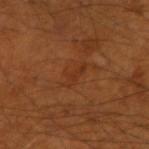* workup — catalogued during a skin exam; not biopsied
* automated metrics — an area of roughly 4 mm², an outline eccentricity of about 0.9 (0 = round, 1 = elongated), and two-axis asymmetry of about 0.3; a mean CIELAB color near L≈32 a*≈24 b*≈33 and a normalized lesion–skin contrast near 5; a border-irregularity rating of about 4/10 and a within-lesion color-variation index near 0/10
* illumination — cross-polarized
* body site — the right forearm
* image source — ~15 mm crop, total-body skin-cancer survey
* lesion diameter — ~3.5 mm (longest diameter)
* subject — male, aged 53 to 57